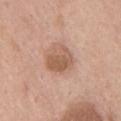No biopsy was performed on this lesion — it was imaged during a full skin examination and was not determined to be concerning.
The patient is a male in their mid-70s.
On the chest.
A 15 mm close-up extracted from a 3D total-body photography capture.
Captured under white-light illumination.